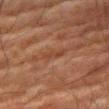The lesion was tiled from a total-body skin photograph and was not biopsied.
Cropped from a total-body skin-imaging series; the visible field is about 15 mm.
Captured under cross-polarized illumination.
Longest diameter approximately 3 mm.
The lesion is on the leg.
The patient is a male aged 78 to 82.
The total-body-photography lesion software estimated a lesion area of about 2 mm², a shape eccentricity near 0.95, and two-axis asymmetry of about 0.4. And it measured internal color variation of about 0 on a 0–10 scale and peripheral color asymmetry of about 0. The analysis additionally found an automated nevus-likeness rating near 0 out of 100 and a lesion-detection confidence of about 55/100.